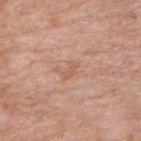Clinical impression: No biopsy was performed on this lesion — it was imaged during a full skin examination and was not determined to be concerning. Background: Longest diameter approximately 2.5 mm. Automated image analysis of the tile measured a footprint of about 3 mm², an outline eccentricity of about 0.85 (0 = round, 1 = elongated), and two-axis asymmetry of about 0.35. From the right upper arm. A lesion tile, about 15 mm wide, cut from a 3D total-body photograph. The subject is a female aged approximately 70. Captured under white-light illumination.